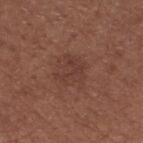Q: Is there a histopathology result?
A: imaged on a skin check; not biopsied
Q: How was the tile lit?
A: white-light
Q: Where on the body is the lesion?
A: the right thigh
Q: Who is the patient?
A: female, aged 53 to 57
Q: What is the imaging modality?
A: ~15 mm tile from a whole-body skin photo